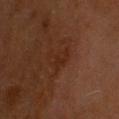Captured during whole-body skin photography for melanoma surveillance; the lesion was not biopsied.
Approximately 3.5 mm at its widest.
The lesion is located on the head or neck.
Imaged with cross-polarized lighting.
A male subject about 60 years old.
A 15 mm crop from a total-body photograph taken for skin-cancer surveillance.
Automated image analysis of the tile measured an area of roughly 4.5 mm², a shape eccentricity near 0.75, and a shape-asymmetry score of about 0.4 (0 = symmetric). And it measured a border-irregularity rating of about 5/10, a within-lesion color-variation index near 2/10, and radial color variation of about 0.5.